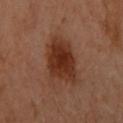notes = total-body-photography surveillance lesion; no biopsy | location = the arm | illumination = cross-polarized | patient = female, about 60 years old | diameter = ~5 mm (longest diameter) | imaging modality = ~15 mm tile from a whole-body skin photo.